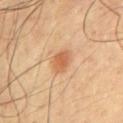<record>
<biopsy_status>not biopsied; imaged during a skin examination</biopsy_status>
<site>chest</site>
<image>
  <source>total-body photography crop</source>
  <field_of_view_mm>15</field_of_view_mm>
</image>
<automated_metrics>
  <area_mm2_approx>5.0</area_mm2_approx>
  <eccentricity>0.7</eccentricity>
  <shape_asymmetry>0.2</shape_asymmetry>
  <cielab_L>61</cielab_L>
  <cielab_a>24</cielab_a>
  <cielab_b>39</cielab_b>
  <vs_skin_darker_L>11.0</vs_skin_darker_L>
  <vs_skin_contrast_norm>7.0</vs_skin_contrast_norm>
  <border_irregularity_0_10>2.0</border_irregularity_0_10>
  <color_variation_0_10>3.5</color_variation_0_10>
  <peripheral_color_asymmetry>1.0</peripheral_color_asymmetry>
</automated_metrics>
<lesion_size>
  <long_diameter_mm_approx>3.0</long_diameter_mm_approx>
</lesion_size>
<lighting>cross-polarized</lighting>
<patient>
  <sex>male</sex>
  <age_approx>65</age_approx>
</patient>
</record>